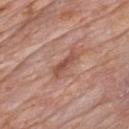Clinical impression:
Imaged during a routine full-body skin examination; the lesion was not biopsied and no histopathology is available.
Image and clinical context:
A 15 mm close-up extracted from a 3D total-body photography capture. Captured under white-light illumination. Located on the chest. The lesion-visualizer software estimated a footprint of about 4.5 mm² and an eccentricity of roughly 0.95. And it measured a detector confidence of about 95 out of 100 that the crop contains a lesion. The patient is a male aged approximately 60. The recorded lesion diameter is about 4 mm.